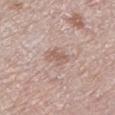Impression:
The lesion was tiled from a total-body skin photograph and was not biopsied.
Background:
A female patient, approximately 65 years of age. About 3 mm across. On the right lower leg. Automated image analysis of the tile measured about 8 CIELAB-L* units darker than the surrounding skin and a normalized lesion–skin contrast near 6. The software also gave border irregularity of about 3 on a 0–10 scale and peripheral color asymmetry of about 0.5. It also reported an automated nevus-likeness rating near 0 out of 100 and lesion-presence confidence of about 100/100. Captured under white-light illumination. A roughly 15 mm field-of-view crop from a total-body skin photograph.